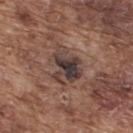Captured during whole-body skin photography for melanoma surveillance; the lesion was not biopsied. The lesion is on the upper back. This image is a 15 mm lesion crop taken from a total-body photograph. Imaged with white-light lighting. A male patient in their mid-70s.